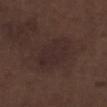Q: Was this lesion biopsied?
A: no biopsy performed (imaged during a skin exam)
Q: What is the lesion's diameter?
A: ≈5.5 mm
Q: Patient demographics?
A: male, approximately 70 years of age
Q: Where on the body is the lesion?
A: the left lower leg
Q: Illumination type?
A: white-light illumination
Q: What kind of image is this?
A: ~15 mm tile from a whole-body skin photo
Q: What did automated image analysis measure?
A: an average lesion color of about L≈26 a*≈15 b*≈16 (CIELAB), roughly 4 lightness units darker than nearby skin, and a lesion-to-skin contrast of about 5.5 (normalized; higher = more distinct); border irregularity of about 2.5 on a 0–10 scale, a within-lesion color-variation index near 2.5/10, and a peripheral color-asymmetry measure near 1